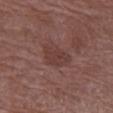Recorded during total-body skin imaging; not selected for excision or biopsy. Located on the left forearm. A lesion tile, about 15 mm wide, cut from a 3D total-body photograph. Automated image analysis of the tile measured a footprint of about 8 mm², a shape eccentricity near 0.7, and a shape-asymmetry score of about 0.25 (0 = symmetric). The analysis additionally found roughly 6 lightness units darker than nearby skin and a normalized border contrast of about 5.5. The software also gave a classifier nevus-likeness of about 0/100 and a detector confidence of about 100 out of 100 that the crop contains a lesion. A female patient, aged 68 to 72. Captured under white-light illumination.